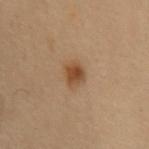- follow-up: catalogued during a skin exam; not biopsied
- lesion size: about 3 mm
- TBP lesion metrics: a symmetry-axis asymmetry near 0.2; border irregularity of about 1.5 on a 0–10 scale, a color-variation rating of about 3/10, and peripheral color asymmetry of about 1
- imaging modality: 15 mm crop, total-body photography
- location: the front of the torso
- subject: female, aged 48–52